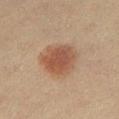No biopsy was performed on this lesion — it was imaged during a full skin examination and was not determined to be concerning.
Imaged with cross-polarized lighting.
Longest diameter approximately 5 mm.
The total-body-photography lesion software estimated a shape eccentricity near 0.5. The software also gave an average lesion color of about L≈44 a*≈18 b*≈27 (CIELAB), about 10 CIELAB-L* units darker than the surrounding skin, and a normalized border contrast of about 8. It also reported a border-irregularity index near 2/10, a within-lesion color-variation index near 3.5/10, and peripheral color asymmetry of about 1.
A 15 mm close-up tile from a total-body photography series done for melanoma screening.
A female patient, approximately 65 years of age.
The lesion is located on the chest.